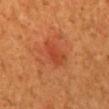Q: Was a biopsy performed?
A: total-body-photography surveillance lesion; no biopsy
Q: What did automated image analysis measure?
A: an eccentricity of roughly 0.9 and a symmetry-axis asymmetry near 0.25; an average lesion color of about L≈44 a*≈33 b*≈38 (CIELAB), about 7 CIELAB-L* units darker than the surrounding skin, and a normalized border contrast of about 5.5; a border-irregularity index near 3/10, a within-lesion color-variation index near 2/10, and radial color variation of about 0.5
Q: What lighting was used for the tile?
A: cross-polarized
Q: What are the patient's age and sex?
A: male, roughly 45 years of age
Q: How was this image acquired?
A: 15 mm crop, total-body photography
Q: Lesion size?
A: ~4 mm (longest diameter)
Q: Where on the body is the lesion?
A: the mid back